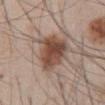The lesion was tiled from a total-body skin photograph and was not biopsied. Located on the abdomen. Longest diameter approximately 5.5 mm. This is a white-light tile. The total-body-photography lesion software estimated a lesion color around L≈48 a*≈18 b*≈26 in CIELAB, about 13 CIELAB-L* units darker than the surrounding skin, and a normalized border contrast of about 10. A male subject about 45 years old. A region of skin cropped from a whole-body photographic capture, roughly 15 mm wide.